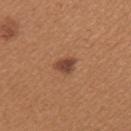Q: Is there a histopathology result?
A: catalogued during a skin exam; not biopsied
Q: What are the patient's age and sex?
A: female, aged 38–42
Q: Automated lesion metrics?
A: a lesion–skin lightness drop of about 11 and a lesion-to-skin contrast of about 9 (normalized; higher = more distinct); an automated nevus-likeness rating near 85 out of 100
Q: How was this image acquired?
A: ~15 mm crop, total-body skin-cancer survey
Q: Lesion size?
A: ~2.5 mm (longest diameter)
Q: How was the tile lit?
A: white-light
Q: What is the anatomic site?
A: the left upper arm